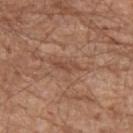- biopsy status: total-body-photography surveillance lesion; no biopsy
- location: the right upper arm
- imaging modality: ~15 mm crop, total-body skin-cancer survey
- tile lighting: white-light illumination
- subject: male, in their mid- to late 60s
- diameter: about 3 mm